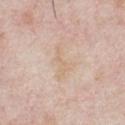Assessment:
Captured during whole-body skin photography for melanoma surveillance; the lesion was not biopsied.
Context:
The recorded lesion diameter is about 4.5 mm. A male subject aged 23–27. From the chest. The tile uses white-light illumination. An algorithmic analysis of the crop reported a lesion–skin lightness drop of about 5 and a normalized border contrast of about 4.5. A 15 mm close-up tile from a total-body photography series done for melanoma screening.